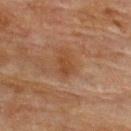Context: A 15 mm close-up extracted from a 3D total-body photography capture. The lesion is located on the upper back. A female patient about 80 years old.